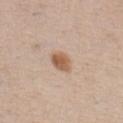{"lesion_size": {"long_diameter_mm_approx": 3.0}, "site": "chest", "image": {"source": "total-body photography crop", "field_of_view_mm": 15}, "patient": {"sex": "male", "age_approx": 50}}